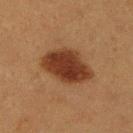Q: Was this lesion biopsied?
A: total-body-photography surveillance lesion; no biopsy
Q: Who is the patient?
A: female, in their 40s
Q: Where on the body is the lesion?
A: the right lower leg
Q: What kind of image is this?
A: ~15 mm tile from a whole-body skin photo
Q: How was the tile lit?
A: cross-polarized illumination
Q: Lesion size?
A: ≈6 mm